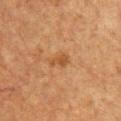A 15 mm close-up extracted from a 3D total-body photography capture. The tile uses cross-polarized illumination. The lesion is on the chest. Automated image analysis of the tile measured a lesion color around L≈40 a*≈19 b*≈32 in CIELAB and a normalized border contrast of about 6.5. It also reported border irregularity of about 4 on a 0–10 scale, a within-lesion color-variation index near 0.5/10, and radial color variation of about 0. And it measured an automated nevus-likeness rating near 15 out of 100 and a lesion-detection confidence of about 100/100. The subject is a male aged around 50. The lesion's longest dimension is about 2.5 mm.